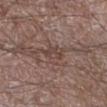{
  "biopsy_status": "not biopsied; imaged during a skin examination",
  "lesion_size": {
    "long_diameter_mm_approx": 3.0
  },
  "patient": {
    "sex": "male",
    "age_approx": 65
  },
  "automated_metrics": {
    "color_variation_0_10": 2.0
  },
  "image": {
    "source": "total-body photography crop",
    "field_of_view_mm": 15
  },
  "site": "right lower leg",
  "lighting": "white-light"
}